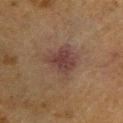Impression:
This lesion was catalogued during total-body skin photography and was not selected for biopsy.
Acquisition and patient details:
Measured at roughly 4.5 mm in maximum diameter. The lesion is located on the left lower leg. This is a cross-polarized tile. A male patient aged 58 to 62. This image is a 15 mm lesion crop taken from a total-body photograph.